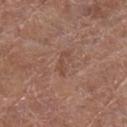workup: total-body-photography surveillance lesion; no biopsy
imaging modality: 15 mm crop, total-body photography
patient: female, roughly 80 years of age
tile lighting: white-light
automated lesion analysis: a lesion-detection confidence of about 100/100
location: the right lower leg
lesion size: ~3.5 mm (longest diameter)